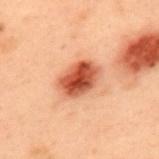The lesion was photographed on a routine skin check and not biopsied; there is no pathology result.
A 15 mm close-up tile from a total-body photography series done for melanoma screening.
Automated tile analysis of the lesion measured a border-irregularity index near 1/10.
On the upper back.
Captured under cross-polarized illumination.
A male patient roughly 55 years of age.